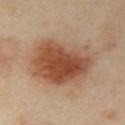The lesion was tiled from a total-body skin photograph and was not biopsied.
Imaged with cross-polarized lighting.
A female subject approximately 40 years of age.
A 15 mm close-up extracted from a 3D total-body photography capture.
From the left arm.
About 8.5 mm across.